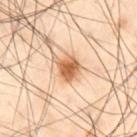Part of a total-body skin-imaging series; this lesion was reviewed on a skin check and was not flagged for biopsy. Imaged with cross-polarized lighting. The lesion is located on the left thigh. Longest diameter approximately 2.5 mm. A roughly 15 mm field-of-view crop from a total-body skin photograph. A male subject aged around 55.